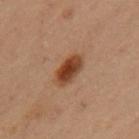Imaged during a routine full-body skin examination; the lesion was not biopsied and no histopathology is available.